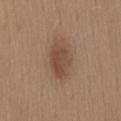Clinical impression:
Part of a total-body skin-imaging series; this lesion was reviewed on a skin check and was not flagged for biopsy.
Background:
The recorded lesion diameter is about 4.5 mm. A roughly 15 mm field-of-view crop from a total-body skin photograph. A female patient, aged approximately 40. The tile uses white-light illumination. On the lower back.